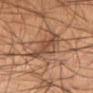The lesion was tiled from a total-body skin photograph and was not biopsied. Located on the leg. The total-body-photography lesion software estimated a lesion area of about 9.5 mm², an eccentricity of roughly 0.6, and two-axis asymmetry of about 0.25. The analysis additionally found a mean CIELAB color near L≈43 a*≈18 b*≈27. A male patient, in their mid-50s. The recorded lesion diameter is about 3.5 mm. Imaged with cross-polarized lighting. Cropped from a total-body skin-imaging series; the visible field is about 15 mm.